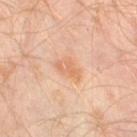Acquisition and patient details:
Automated tile analysis of the lesion measured an area of roughly 5 mm² and an eccentricity of roughly 0.75. The analysis additionally found a lesion color around L≈66 a*≈22 b*≈35 in CIELAB and roughly 7 lightness units darker than nearby skin. The software also gave a border-irregularity rating of about 3/10, a within-lesion color-variation index near 3/10, and peripheral color asymmetry of about 1.5. The software also gave a nevus-likeness score of about 10/100 and a lesion-detection confidence of about 100/100. The lesion is on the left leg. Captured under cross-polarized illumination. The recorded lesion diameter is about 3 mm. Cropped from a total-body skin-imaging series; the visible field is about 15 mm. The patient is a male aged approximately 30.